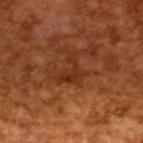Impression:
Recorded during total-body skin imaging; not selected for excision or biopsy.
Image and clinical context:
Cropped from a total-body skin-imaging series; the visible field is about 15 mm. A male patient in their mid-60s.